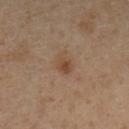Assessment: No biopsy was performed on this lesion — it was imaged during a full skin examination and was not determined to be concerning. Context: A male subject aged 63–67. About 2.5 mm across. Captured under cross-polarized illumination. A 15 mm close-up tile from a total-body photography series done for melanoma screening. From the left lower leg.